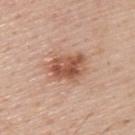workup=imaged on a skin check; not biopsied
automated lesion analysis=about 13 CIELAB-L* units darker than the surrounding skin and a normalized border contrast of about 8.5; an automated nevus-likeness rating near 75 out of 100
size=about 5 mm
patient=male, aged 53–57
imaging modality=~15 mm tile from a whole-body skin photo
site=the upper back